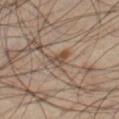Clinical impression: Imaged during a routine full-body skin examination; the lesion was not biopsied and no histopathology is available. Background: A 15 mm close-up tile from a total-body photography series done for melanoma screening. The lesion is located on the right thigh. A male subject aged 58 to 62. Longest diameter approximately 2.5 mm. Automated tile analysis of the lesion measured a footprint of about 3.5 mm², a shape eccentricity near 0.75, and a shape-asymmetry score of about 0.4 (0 = symmetric). The analysis additionally found a lesion color around L≈46 a*≈15 b*≈26 in CIELAB, a lesion–skin lightness drop of about 9, and a normalized border contrast of about 7. It also reported a border-irregularity rating of about 4/10, a color-variation rating of about 4/10, and peripheral color asymmetry of about 1.